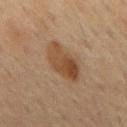No biopsy was performed on this lesion — it was imaged during a full skin examination and was not determined to be concerning. A lesion tile, about 15 mm wide, cut from a 3D total-body photograph. On the back. A male subject, roughly 70 years of age. The recorded lesion diameter is about 5.5 mm.